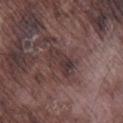<record>
  <biopsy_status>not biopsied; imaged during a skin examination</biopsy_status>
  <patient>
    <sex>male</sex>
    <age_approx>75</age_approx>
  </patient>
  <site>right lower leg</site>
  <image>
    <source>total-body photography crop</source>
    <field_of_view_mm>15</field_of_view_mm>
  </image>
</record>